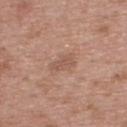Assessment: Recorded during total-body skin imaging; not selected for excision or biopsy. Image and clinical context: A male patient, in their mid- to late 60s. Approximately 3 mm at its widest. This is a white-light tile. A 15 mm crop from a total-body photograph taken for skin-cancer surveillance. From the upper back.